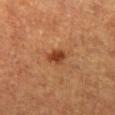follow-up = imaged on a skin check; not biopsied | TBP lesion metrics = a color-variation rating of about 2/10 and a peripheral color-asymmetry measure near 0.5 | body site = the left thigh | acquisition = total-body-photography crop, ~15 mm field of view | subject = male, approximately 60 years of age | diameter = ~2.5 mm (longest diameter).